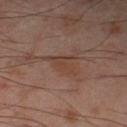Recorded during total-body skin imaging; not selected for excision or biopsy.
Captured under cross-polarized illumination.
A 15 mm close-up tile from a total-body photography series done for melanoma screening.
Measured at roughly 4 mm in maximum diameter.
The lesion is on the left thigh.
A male subject roughly 55 years of age.